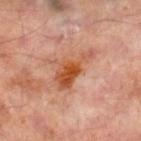{
  "image": {
    "source": "total-body photography crop",
    "field_of_view_mm": 15
  },
  "site": "right lower leg",
  "patient": {
    "sex": "male",
    "age_approx": 65
  }
}